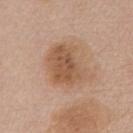This is a white-light tile. A female patient aged 68 to 72. About 6 mm across. Automated tile analysis of the lesion measured a border-irregularity index near 3.5/10 and a color-variation rating of about 4.5/10. A 15 mm crop from a total-body photograph taken for skin-cancer surveillance. The lesion is located on the front of the torso.